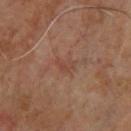| key | value |
|---|---|
| biopsy status | total-body-photography surveillance lesion; no biopsy |
| image source | ~15 mm crop, total-body skin-cancer survey |
| subject | male, approximately 65 years of age |
| site | the back |